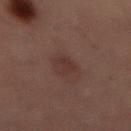workup: imaged on a skin check; not biopsied | image source: total-body-photography crop, ~15 mm field of view | patient: male, aged 53–57 | location: the abdomen | illumination: cross-polarized | lesion size: ~3 mm (longest diameter).